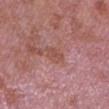notes: catalogued during a skin exam; not biopsied
subject: female, approximately 40 years of age
image source: ~15 mm tile from a whole-body skin photo
body site: the arm
lesion size: about 3 mm